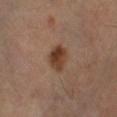Imaged during a routine full-body skin examination; the lesion was not biopsied and no histopathology is available.